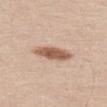Q: Was a biopsy performed?
A: no biopsy performed (imaged during a skin exam)
Q: What is the imaging modality?
A: 15 mm crop, total-body photography
Q: Who is the patient?
A: male, roughly 60 years of age
Q: Automated lesion metrics?
A: an area of roughly 9 mm² and a shape-asymmetry score of about 0.15 (0 = symmetric); an automated nevus-likeness rating near 95 out of 100 and a detector confidence of about 100 out of 100 that the crop contains a lesion
Q: Where on the body is the lesion?
A: the left thigh